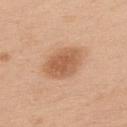| key | value |
|---|---|
| workup | catalogued during a skin exam; not biopsied |
| diameter | ~5 mm (longest diameter) |
| lighting | white-light |
| automated lesion analysis | a lesion area of about 13 mm² and a shape-asymmetry score of about 0.15 (0 = symmetric); a lesion color around L≈59 a*≈22 b*≈35 in CIELAB and a normalized border contrast of about 7.5; a classifier nevus-likeness of about 90/100 and lesion-presence confidence of about 100/100 |
| subject | female, approximately 40 years of age |
| body site | the upper back |
| acquisition | ~15 mm tile from a whole-body skin photo |